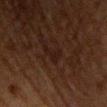Captured during whole-body skin photography for melanoma surveillance; the lesion was not biopsied. Located on the chest. A roughly 15 mm field-of-view crop from a total-body skin photograph. A male subject aged 63 to 67. This is a cross-polarized tile. Automated image analysis of the tile measured an automated nevus-likeness rating near 0 out of 100 and lesion-presence confidence of about 90/100.